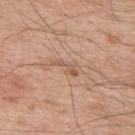Findings:
• follow-up: no biopsy performed (imaged during a skin exam)
• anatomic site: the upper back
• image source: total-body-photography crop, ~15 mm field of view
• patient: male, aged 53 to 57